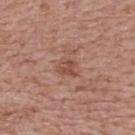Clinical impression:
No biopsy was performed on this lesion — it was imaged during a full skin examination and was not determined to be concerning.
Acquisition and patient details:
Captured under white-light illumination. A 15 mm close-up tile from a total-body photography series done for melanoma screening. Located on the upper back. A female subject, aged 63 to 67.